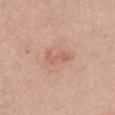No biopsy was performed on this lesion — it was imaged during a full skin examination and was not determined to be concerning.
Cropped from a whole-body photographic skin survey; the tile spans about 15 mm.
Located on the chest.
Approximately 3 mm at its widest.
A female patient, aged around 45.
Automated image analysis of the tile measured an outline eccentricity of about 0.9 (0 = round, 1 = elongated) and a shape-asymmetry score of about 0.45 (0 = symmetric). And it measured a mean CIELAB color near L≈60 a*≈23 b*≈29 and about 8 CIELAB-L* units darker than the surrounding skin. It also reported an automated nevus-likeness rating near 0 out of 100 and a lesion-detection confidence of about 100/100.